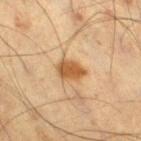  biopsy_status: not biopsied; imaged during a skin examination
  automated_metrics:
    area_mm2_approx: 6.5
    eccentricity: 0.7
    shape_asymmetry: 0.15
    cielab_L: 47
    cielab_a: 18
    cielab_b: 35
    vs_skin_darker_L: 12.0
    vs_skin_contrast_norm: 9.5
    border_irregularity_0_10: 1.5
    color_variation_0_10: 2.0
    peripheral_color_asymmetry: 0.5
  image:
    source: total-body photography crop
    field_of_view_mm: 15
  lighting: cross-polarized
  site: right thigh
  lesion_size:
    long_diameter_mm_approx: 3.5
  patient:
    sex: male
    age_approx: 60